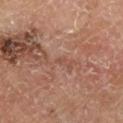Recorded during total-body skin imaging; not selected for excision or biopsy. About 1.5 mm across. A male subject approximately 65 years of age. From the right lower leg. Imaged with cross-polarized lighting. Cropped from a total-body skin-imaging series; the visible field is about 15 mm.